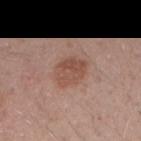Assessment: Recorded during total-body skin imaging; not selected for excision or biopsy. Context: A lesion tile, about 15 mm wide, cut from a 3D total-body photograph. Automated image analysis of the tile measured a lesion area of about 9 mm², an outline eccentricity of about 0.65 (0 = round, 1 = elongated), and a shape-asymmetry score of about 0.2 (0 = symmetric). And it measured a border-irregularity index near 2/10. The lesion's longest dimension is about 4 mm. A male patient, aged 28–32. The lesion is on the arm.